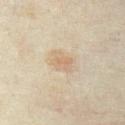Case summary:
* follow-up — imaged on a skin check; not biopsied
* tile lighting — cross-polarized illumination
* image — 15 mm crop, total-body photography
* body site — the abdomen
* patient — female, in their mid-30s
* automated lesion analysis — an area of roughly 5.5 mm² and a symmetry-axis asymmetry near 0.2